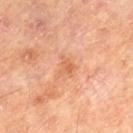Impression:
Recorded during total-body skin imaging; not selected for excision or biopsy.
Image and clinical context:
Automated tile analysis of the lesion measured a footprint of about 3.5 mm², a shape eccentricity near 0.6, and two-axis asymmetry of about 0.35. It also reported a lesion color around L≈60 a*≈26 b*≈38 in CIELAB, a lesion–skin lightness drop of about 7, and a lesion-to-skin contrast of about 5.5 (normalized; higher = more distinct). It also reported border irregularity of about 4 on a 0–10 scale, a color-variation rating of about 2/10, and radial color variation of about 0.5. It also reported lesion-presence confidence of about 100/100. Longest diameter approximately 2.5 mm. Imaged with cross-polarized lighting. A 15 mm close-up extracted from a 3D total-body photography capture. The lesion is on the left thigh. The subject is a male approximately 65 years of age.